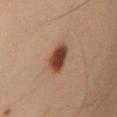Imaged during a routine full-body skin examination; the lesion was not biopsied and no histopathology is available. A male subject about 55 years old. A 15 mm close-up extracted from a 3D total-body photography capture. From the arm. Captured under cross-polarized illumination.